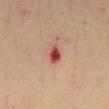Imaged during a routine full-body skin examination; the lesion was not biopsied and no histopathology is available. Captured under cross-polarized illumination. A male patient roughly 30 years of age. A 15 mm close-up tile from a total-body photography series done for melanoma screening. Located on the abdomen. The recorded lesion diameter is about 2.5 mm.